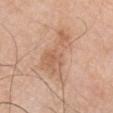No biopsy was performed on this lesion — it was imaged during a full skin examination and was not determined to be concerning. Longest diameter approximately 6.5 mm. A male patient in their mid-70s. Automated tile analysis of the lesion measured a footprint of about 16 mm², an outline eccentricity of about 0.85 (0 = round, 1 = elongated), and a shape-asymmetry score of about 0.55 (0 = symmetric). The software also gave a lesion color around L≈61 a*≈20 b*≈32 in CIELAB, roughly 8 lightness units darker than nearby skin, and a normalized border contrast of about 6. And it measured border irregularity of about 6 on a 0–10 scale, internal color variation of about 3.5 on a 0–10 scale, and radial color variation of about 1. Imaged with white-light lighting. A 15 mm crop from a total-body photograph taken for skin-cancer surveillance. The lesion is on the right upper arm.